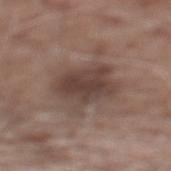Image and clinical context: Located on the back. This image is a 15 mm lesion crop taken from a total-body photograph. The tile uses white-light illumination. A male patient aged 58 to 62. The lesion's longest dimension is about 6 mm.